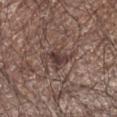No biopsy was performed on this lesion — it was imaged during a full skin examination and was not determined to be concerning.
A male patient about 65 years old.
From the left upper arm.
This image is a 15 mm lesion crop taken from a total-body photograph.
The lesion's longest dimension is about 3.5 mm.
Imaged with white-light lighting.